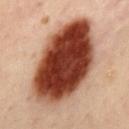| feature | finding |
|---|---|
| acquisition | 15 mm crop, total-body photography |
| patient | male, approximately 50 years of age |
| automated lesion analysis | an average lesion color of about L≈35 a*≈22 b*≈26 (CIELAB), roughly 22 lightness units darker than nearby skin, and a normalized lesion–skin contrast near 17; a border-irregularity rating of about 1.5/10, a within-lesion color-variation index near 10/10, and radial color variation of about 3 |
| location | the mid back |
| illumination | cross-polarized illumination |